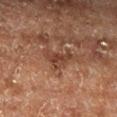Clinical summary:
The patient is a male approximately 75 years of age. An algorithmic analysis of the crop reported a shape eccentricity near 0.9. And it measured a mean CIELAB color near L≈32 a*≈18 b*≈24, roughly 7 lightness units darker than nearby skin, and a normalized border contrast of about 7. The software also gave a border-irregularity index near 6/10, a within-lesion color-variation index near 2.5/10, and peripheral color asymmetry of about 0.5. It also reported an automated nevus-likeness rating near 0 out of 100. This image is a 15 mm lesion crop taken from a total-body photograph. The lesion is on the left lower leg. The recorded lesion diameter is about 4.5 mm.